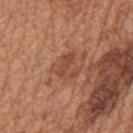Part of a total-body skin-imaging series; this lesion was reviewed on a skin check and was not flagged for biopsy. A male patient, in their mid- to late 60s. The lesion is located on the mid back. Imaged with white-light lighting. About 4 mm across. A 15 mm close-up tile from a total-body photography series done for melanoma screening.